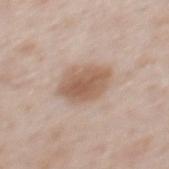The lesion was tiled from a total-body skin photograph and was not biopsied.
The lesion's longest dimension is about 5 mm.
A male subject, approximately 40 years of age.
A roughly 15 mm field-of-view crop from a total-body skin photograph.
The tile uses white-light illumination.
Located on the mid back.
The lesion-visualizer software estimated a mean CIELAB color near L≈58 a*≈17 b*≈28, roughly 11 lightness units darker than nearby skin, and a normalized border contrast of about 8. It also reported a nevus-likeness score of about 95/100.